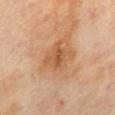The lesion was tiled from a total-body skin photograph and was not biopsied. An algorithmic analysis of the crop reported a footprint of about 8 mm², a shape eccentricity near 0.45, and a symmetry-axis asymmetry near 0.25. The analysis additionally found a lesion color around L≈58 a*≈23 b*≈38 in CIELAB and a normalized lesion–skin contrast near 6. The software also gave a nevus-likeness score of about 0/100 and a lesion-detection confidence of about 100/100. The recorded lesion diameter is about 3.5 mm. A roughly 15 mm field-of-view crop from a total-body skin photograph. The subject is a female aged 58–62. The lesion is located on the mid back.